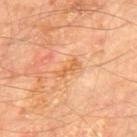anatomic site — the mid back
diameter — about 3 mm
subject — male, aged 63–67
lighting — cross-polarized
automated metrics — an outline eccentricity of about 0.95 (0 = round, 1 = elongated)
image — total-body-photography crop, ~15 mm field of view